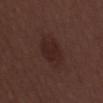Q: Is there a histopathology result?
A: imaged on a skin check; not biopsied
Q: What are the patient's age and sex?
A: male, roughly 30 years of age
Q: Illumination type?
A: white-light illumination
Q: Lesion location?
A: the mid back
Q: How was this image acquired?
A: ~15 mm tile from a whole-body skin photo
Q: What is the lesion's diameter?
A: about 4 mm